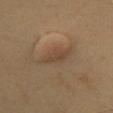biopsy status=total-body-photography surveillance lesion; no biopsy | tile lighting=cross-polarized | patient=male, in their 50s | site=the chest | automated lesion analysis=an area of roughly 6.5 mm², an eccentricity of roughly 0.85, and a symmetry-axis asymmetry near 0.25; a classifier nevus-likeness of about 80/100 and a lesion-detection confidence of about 100/100 | diameter=≈4.5 mm | imaging modality=15 mm crop, total-body photography.